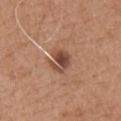Q: Who is the patient?
A: male, in their mid- to late 60s
Q: What kind of image is this?
A: 15 mm crop, total-body photography
Q: What is the lesion's diameter?
A: ≈2.5 mm
Q: What did automated image analysis measure?
A: an area of roughly 6 mm² and a shape eccentricity near 0.45; a classifier nevus-likeness of about 15/100
Q: Lesion location?
A: the front of the torso